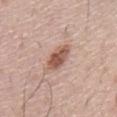notes: no biopsy performed (imaged during a skin exam) | subject: male, aged around 75 | site: the chest | illumination: white-light illumination | imaging modality: ~15 mm tile from a whole-body skin photo | TBP lesion metrics: an average lesion color of about L≈54 a*≈22 b*≈27 (CIELAB), about 14 CIELAB-L* units darker than the surrounding skin, and a lesion-to-skin contrast of about 9.5 (normalized; higher = more distinct); lesion-presence confidence of about 100/100.